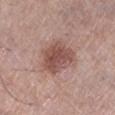The lesion was tiled from a total-body skin photograph and was not biopsied. A close-up tile cropped from a whole-body skin photograph, about 15 mm across. A female subject aged around 70. On the left lower leg.